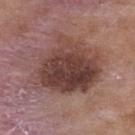follow-up=imaged on a skin check; not biopsied | imaging modality=~15 mm crop, total-body skin-cancer survey | TBP lesion metrics=a border-irregularity rating of about 3.5/10 and radial color variation of about 2.5 | tile lighting=white-light illumination | lesion size=≈7.5 mm | patient=female, aged around 40 | anatomic site=the upper back.